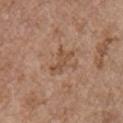<tbp_lesion>
  <biopsy_status>not biopsied; imaged during a skin examination</biopsy_status>
  <patient>
    <sex>female</sex>
    <age_approx>65</age_approx>
  </patient>
  <lesion_size>
    <long_diameter_mm_approx>4.0</long_diameter_mm_approx>
  </lesion_size>
  <automated_metrics>
    <area_mm2_approx>5.0</area_mm2_approx>
    <eccentricity>0.9</eccentricity>
    <shape_asymmetry>0.4</shape_asymmetry>
    <border_irregularity_0_10>5.0</border_irregularity_0_10>
  </automated_metrics>
  <site>right upper arm</site>
  <image>
    <source>total-body photography crop</source>
    <field_of_view_mm>15</field_of_view_mm>
  </image>
</tbp_lesion>